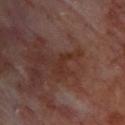Clinical impression:
No biopsy was performed on this lesion — it was imaged during a full skin examination and was not determined to be concerning.
Background:
A male subject, aged 68 to 72. Imaged with cross-polarized lighting. An algorithmic analysis of the crop reported a footprint of about 2.5 mm², an outline eccentricity of about 0.85 (0 = round, 1 = elongated), and a symmetry-axis asymmetry near 0.55. On the upper back. About 2.5 mm across. A close-up tile cropped from a whole-body skin photograph, about 15 mm across.